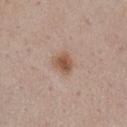Case summary:
* follow-up — imaged on a skin check; not biopsied
* illumination — white-light illumination
* patient — female, approximately 55 years of age
* imaging modality — 15 mm crop, total-body photography
* site — the chest
* diameter — about 3 mm